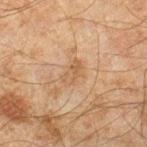Recorded during total-body skin imaging; not selected for excision or biopsy.
A male subject, in their mid- to late 40s.
From the right lower leg.
Cropped from a whole-body photographic skin survey; the tile spans about 15 mm.
This is a cross-polarized tile.
About 3 mm across.